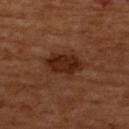  image:
    source: total-body photography crop
    field_of_view_mm: 15
  patient:
    sex: male
    age_approx: 65
  site: upper back